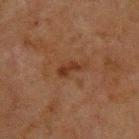| key | value |
|---|---|
| workup | total-body-photography surveillance lesion; no biopsy |
| image | ~15 mm crop, total-body skin-cancer survey |
| subject | male, in their 60s |
| location | the upper back |